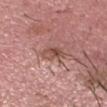Impression:
Part of a total-body skin-imaging series; this lesion was reviewed on a skin check and was not flagged for biopsy.
Context:
The total-body-photography lesion software estimated a lesion area of about 4.5 mm², an outline eccentricity of about 0.8 (0 = round, 1 = elongated), and two-axis asymmetry of about 0.25. The software also gave an average lesion color of about L≈50 a*≈22 b*≈25 (CIELAB) and a lesion–skin lightness drop of about 9. It also reported a nevus-likeness score of about 10/100 and a lesion-detection confidence of about 100/100. This image is a 15 mm lesion crop taken from a total-body photograph. A male subject aged around 30. This is a white-light tile. The lesion's longest dimension is about 3 mm. Located on the head or neck.